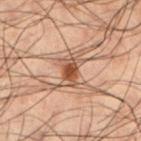Recorded during total-body skin imaging; not selected for excision or biopsy.
The recorded lesion diameter is about 3 mm.
A male patient, roughly 50 years of age.
A 15 mm crop from a total-body photograph taken for skin-cancer surveillance.
The lesion is located on the left lower leg.
This is a cross-polarized tile.